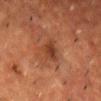Part of a total-body skin-imaging series; this lesion was reviewed on a skin check and was not flagged for biopsy.